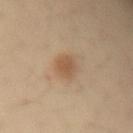The lesion was tiled from a total-body skin photograph and was not biopsied.
An algorithmic analysis of the crop reported a lesion color around L≈54 a*≈17 b*≈32 in CIELAB. And it measured a border-irregularity index near 2.5/10, internal color variation of about 2.5 on a 0–10 scale, and peripheral color asymmetry of about 1.
A female patient roughly 35 years of age.
From the left forearm.
A 15 mm close-up tile from a total-body photography series done for melanoma screening.
The recorded lesion diameter is about 3.5 mm.
This is a cross-polarized tile.